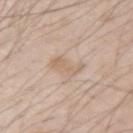lighting — white-light
site — the arm
diameter — ≈4 mm
subject — male, in their mid-40s
image source — total-body-photography crop, ~15 mm field of view